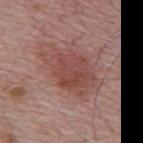workup: total-body-photography surveillance lesion; no biopsy | patient: male, aged 63 to 67 | lesion size: about 6.5 mm | imaging modality: 15 mm crop, total-body photography | automated metrics: an average lesion color of about L≈47 a*≈24 b*≈24 (CIELAB) and a normalized border contrast of about 7; a border-irregularity index near 3.5/10, internal color variation of about 3.5 on a 0–10 scale, and peripheral color asymmetry of about 1 | anatomic site: the upper back.